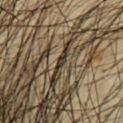Case summary:
* workup · total-body-photography surveillance lesion; no biopsy